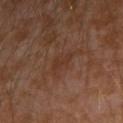Part of a total-body skin-imaging series; this lesion was reviewed on a skin check and was not flagged for biopsy.
This is a cross-polarized tile.
A lesion tile, about 15 mm wide, cut from a 3D total-body photograph.
A male patient roughly 30 years of age.
The total-body-photography lesion software estimated a footprint of about 3.5 mm², a shape eccentricity near 0.8, and a shape-asymmetry score of about 0.35 (0 = symmetric). The analysis additionally found a mean CIELAB color near L≈32 a*≈19 b*≈26, roughly 4 lightness units darker than nearby skin, and a normalized border contrast of about 5.
From the left arm.
Measured at roughly 3 mm in maximum diameter.